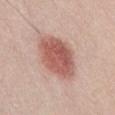This lesion was catalogued during total-body skin photography and was not selected for biopsy. The total-body-photography lesion software estimated an area of roughly 19 mm² and an eccentricity of roughly 0.75. The software also gave a border-irregularity index near 1.5/10, internal color variation of about 4.5 on a 0–10 scale, and peripheral color asymmetry of about 1.5. The analysis additionally found lesion-presence confidence of about 100/100. A close-up tile cropped from a whole-body skin photograph, about 15 mm across. A male subject, about 25 years old. The lesion is on the abdomen.